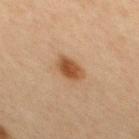Q: Was this lesion biopsied?
A: imaged on a skin check; not biopsied
Q: What kind of image is this?
A: ~15 mm crop, total-body skin-cancer survey
Q: Automated lesion metrics?
A: an outline eccentricity of about 0.55 (0 = round, 1 = elongated) and a shape-asymmetry score of about 0.2 (0 = symmetric)
Q: Patient demographics?
A: female, aged approximately 45
Q: What lighting was used for the tile?
A: cross-polarized illumination
Q: How large is the lesion?
A: ~3 mm (longest diameter)
Q: Where on the body is the lesion?
A: the upper back